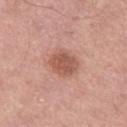Clinical impression:
Captured during whole-body skin photography for melanoma surveillance; the lesion was not biopsied.
Background:
A male subject roughly 70 years of age. The lesion is on the left thigh. A region of skin cropped from a whole-body photographic capture, roughly 15 mm wide. The tile uses white-light illumination. Longest diameter approximately 3.5 mm.